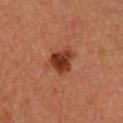Assessment:
The lesion was tiled from a total-body skin photograph and was not biopsied.
Clinical summary:
This is a cross-polarized tile. From the right forearm. The subject is a female approximately 35 years of age. Cropped from a whole-body photographic skin survey; the tile spans about 15 mm. Automated image analysis of the tile measured an average lesion color of about L≈37 a*≈28 b*≈32 (CIELAB). The software also gave a classifier nevus-likeness of about 100/100. Longest diameter approximately 3.5 mm.